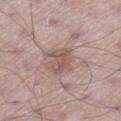| field | value |
|---|---|
| notes | imaged on a skin check; not biopsied |
| image source | ~15 mm crop, total-body skin-cancer survey |
| anatomic site | the left thigh |
| subject | male, in their mid- to late 60s |